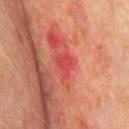Impression: Captured during whole-body skin photography for melanoma surveillance; the lesion was not biopsied. Clinical summary: A male patient, about 75 years old. Measured at roughly 2.5 mm in maximum diameter. A close-up tile cropped from a whole-body skin photograph, about 15 mm across. Imaged with cross-polarized lighting. The lesion is on the chest.